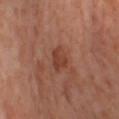follow-up: catalogued during a skin exam; not biopsied | illumination: cross-polarized | subject: female, aged 53 to 57 | lesion size: ≈3 mm | automated lesion analysis: an area of roughly 5.5 mm² and an outline eccentricity of about 0.55 (0 = round, 1 = elongated); a mean CIELAB color near L≈42 a*≈25 b*≈30 and about 8 CIELAB-L* units darker than the surrounding skin; border irregularity of about 2 on a 0–10 scale, a color-variation rating of about 2.5/10, and radial color variation of about 1; an automated nevus-likeness rating near 5 out of 100 and a detector confidence of about 100 out of 100 that the crop contains a lesion | site: the left thigh | imaging modality: 15 mm crop, total-body photography.